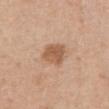workup: imaged on a skin check; not biopsied
patient: female, in their 40s
location: the abdomen
automated lesion analysis: roughly 11 lightness units darker than nearby skin and a lesion-to-skin contrast of about 7.5 (normalized; higher = more distinct); a border-irregularity index near 2.5/10, internal color variation of about 3 on a 0–10 scale, and peripheral color asymmetry of about 1; a nevus-likeness score of about 25/100 and a lesion-detection confidence of about 100/100
tile lighting: white-light illumination
lesion diameter: ~3.5 mm (longest diameter)
image: total-body-photography crop, ~15 mm field of view